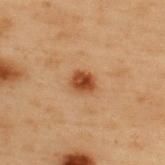A 15 mm close-up tile from a total-body photography series done for melanoma screening.
The patient is a male in their mid-50s.
Captured under cross-polarized illumination.
The lesion is located on the back.
Automated tile analysis of the lesion measured a lesion area of about 5 mm² and a symmetry-axis asymmetry near 0.2. And it measured border irregularity of about 2 on a 0–10 scale, a within-lesion color-variation index near 3.5/10, and a peripheral color-asymmetry measure near 1.5. The analysis additionally found a nevus-likeness score of about 95/100 and a detector confidence of about 100 out of 100 that the crop contains a lesion.
The lesion's longest dimension is about 2.5 mm.